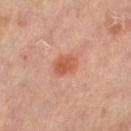Impression: This lesion was catalogued during total-body skin photography and was not selected for biopsy. Clinical summary: About 3 mm across. A 15 mm close-up tile from a total-body photography series done for melanoma screening. The lesion is on the right leg. The patient is a female aged around 65. Automated image analysis of the tile measured a classifier nevus-likeness of about 95/100 and a lesion-detection confidence of about 100/100.